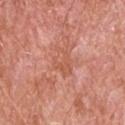<case>
  <biopsy_status>not biopsied; imaged during a skin examination</biopsy_status>
  <image>
    <source>total-body photography crop</source>
    <field_of_view_mm>15</field_of_view_mm>
  </image>
  <site>head or neck</site>
  <lesion_size>
    <long_diameter_mm_approx>3.5</long_diameter_mm_approx>
  </lesion_size>
  <patient>
    <sex>male</sex>
    <age_approx>80</age_approx>
  </patient>
</case>